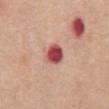Recorded during total-body skin imaging; not selected for excision or biopsy.
The lesion is located on the front of the torso.
A male patient, in their mid- to late 60s.
The recorded lesion diameter is about 3 mm.
A close-up tile cropped from a whole-body skin photograph, about 15 mm across.